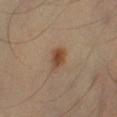Imaged during a routine full-body skin examination; the lesion was not biopsied and no histopathology is available. A region of skin cropped from a whole-body photographic capture, roughly 15 mm wide. Longest diameter approximately 3 mm. The lesion is on the left lower leg. A male patient, approximately 40 years of age. Automated image analysis of the tile measured a lesion color around L≈46 a*≈19 b*≈31 in CIELAB, a lesion–skin lightness drop of about 10, and a normalized lesion–skin contrast near 8.5. The software also gave border irregularity of about 2 on a 0–10 scale and peripheral color asymmetry of about 1.5. The analysis additionally found a classifier nevus-likeness of about 100/100 and a detector confidence of about 100 out of 100 that the crop contains a lesion.